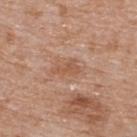| field | value |
|---|---|
| follow-up | total-body-photography surveillance lesion; no biopsy |
| acquisition | total-body-photography crop, ~15 mm field of view |
| subject | female, about 75 years old |
| lesion diameter | about 2.5 mm |
| illumination | white-light |
| anatomic site | the upper back |